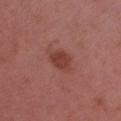No biopsy was performed on this lesion — it was imaged during a full skin examination and was not determined to be concerning. A 15 mm close-up extracted from a 3D total-body photography capture. The lesion's longest dimension is about 3 mm. Automated image analysis of the tile measured an average lesion color of about L≈41 a*≈27 b*≈26 (CIELAB), a lesion–skin lightness drop of about 8, and a normalized border contrast of about 7. The analysis additionally found lesion-presence confidence of about 100/100. A male subject aged 53–57. From the right upper arm. This is a white-light tile.